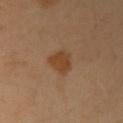Impression:
Recorded during total-body skin imaging; not selected for excision or biopsy.
Clinical summary:
Located on the right upper arm. Automated tile analysis of the lesion measured about 8 CIELAB-L* units darker than the surrounding skin. It also reported an automated nevus-likeness rating near 75 out of 100 and a lesion-detection confidence of about 100/100. A male patient, aged 38 to 42. Imaged with cross-polarized lighting. The lesion's longest dimension is about 3.5 mm. A 15 mm crop from a total-body photograph taken for skin-cancer surveillance.